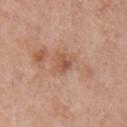Assessment: Captured during whole-body skin photography for melanoma surveillance; the lesion was not biopsied. Acquisition and patient details: An algorithmic analysis of the crop reported an area of roughly 4 mm², a shape eccentricity near 0.5, and a shape-asymmetry score of about 0.3 (0 = symmetric). It also reported an average lesion color of about L≈54 a*≈22 b*≈31 (CIELAB) and a normalized border contrast of about 6.5. It also reported a nevus-likeness score of about 0/100 and a detector confidence of about 100 out of 100 that the crop contains a lesion. Cropped from a whole-body photographic skin survey; the tile spans about 15 mm. The tile uses white-light illumination. About 2.5 mm across. Located on the chest. A male patient aged 68 to 72.